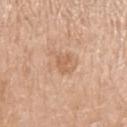Assessment:
Recorded during total-body skin imaging; not selected for excision or biopsy.
Image and clinical context:
The patient is a male aged 68–72. A close-up tile cropped from a whole-body skin photograph, about 15 mm across. On the left upper arm.